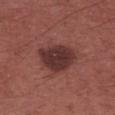Q: Was this lesion biopsied?
A: no biopsy performed (imaged during a skin exam)
Q: Who is the patient?
A: male, aged 53–57
Q: What is the lesion's diameter?
A: about 4.5 mm
Q: Where on the body is the lesion?
A: the left upper arm
Q: Illumination type?
A: white-light
Q: How was this image acquired?
A: ~15 mm crop, total-body skin-cancer survey